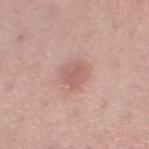follow-up = total-body-photography surveillance lesion; no biopsy | acquisition = 15 mm crop, total-body photography | patient = female, roughly 55 years of age | anatomic site = the left thigh.